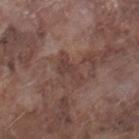No biopsy was performed on this lesion — it was imaged during a full skin examination and was not determined to be concerning. A male patient, aged 73 to 77. Captured under white-light illumination. Located on the leg. The lesion's longest dimension is about 4 mm. An algorithmic analysis of the crop reported an eccentricity of roughly 0.9 and a shape-asymmetry score of about 0.55 (0 = symmetric). A close-up tile cropped from a whole-body skin photograph, about 15 mm across.